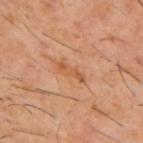The lesion was photographed on a routine skin check and not biopsied; there is no pathology result.
The total-body-photography lesion software estimated a mean CIELAB color near L≈52 a*≈23 b*≈35, a lesion–skin lightness drop of about 7, and a normalized border contrast of about 5.5. The analysis additionally found a nevus-likeness score of about 0/100 and a lesion-detection confidence of about 100/100.
The recorded lesion diameter is about 3.5 mm.
The subject is a male roughly 60 years of age.
The tile uses cross-polarized illumination.
The lesion is on the upper back.
Cropped from a whole-body photographic skin survey; the tile spans about 15 mm.